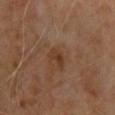Part of a total-body skin-imaging series; this lesion was reviewed on a skin check and was not flagged for biopsy. Measured at roughly 2.5 mm in maximum diameter. Imaged with cross-polarized lighting. The total-body-photography lesion software estimated an area of roughly 3.5 mm², an eccentricity of roughly 0.8, and two-axis asymmetry of about 0.25. The software also gave a lesion color around L≈35 a*≈18 b*≈29 in CIELAB, roughly 7 lightness units darker than nearby skin, and a normalized border contrast of about 7. And it measured a border-irregularity rating of about 3/10, a within-lesion color-variation index near 3/10, and a peripheral color-asymmetry measure near 1. It also reported a classifier nevus-likeness of about 0/100 and a detector confidence of about 100 out of 100 that the crop contains a lesion. A male patient approximately 65 years of age. A lesion tile, about 15 mm wide, cut from a 3D total-body photograph. On the chest.